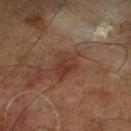<case>
<biopsy_status>not biopsied; imaged during a skin examination</biopsy_status>
<lighting>cross-polarized</lighting>
<patient>
  <sex>male</sex>
  <age_approx>70</age_approx>
</patient>
<lesion_size>
  <long_diameter_mm_approx>4.0</long_diameter_mm_approx>
</lesion_size>
<site>leg</site>
<image>
  <source>total-body photography crop</source>
  <field_of_view_mm>15</field_of_view_mm>
</image>
</case>